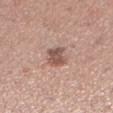Case summary:
– biopsy status — imaged on a skin check; not biopsied
– patient — female, approximately 30 years of age
– automated lesion analysis — a border-irregularity rating of about 2/10, a within-lesion color-variation index near 2.5/10, and peripheral color asymmetry of about 1; a classifier nevus-likeness of about 15/100 and a lesion-detection confidence of about 100/100
– site — the left lower leg
– size — ≈2.5 mm
– tile lighting — white-light
– acquisition — ~15 mm tile from a whole-body skin photo